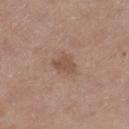Captured during whole-body skin photography for melanoma surveillance; the lesion was not biopsied. A male subject, aged 73 to 77. Located on the leg. The total-body-photography lesion software estimated a classifier nevus-likeness of about 10/100 and a lesion-detection confidence of about 100/100. A 15 mm close-up extracted from a 3D total-body photography capture. The lesion's longest dimension is about 3 mm. Imaged with white-light lighting.